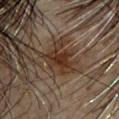{"biopsy_status": "not biopsied; imaged during a skin examination", "site": "head or neck", "lesion_size": {"long_diameter_mm_approx": 2.5}, "image": {"source": "total-body photography crop", "field_of_view_mm": 15}, "patient": {"sex": "female", "age_approx": 45}}